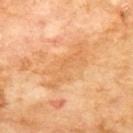Part of a total-body skin-imaging series; this lesion was reviewed on a skin check and was not flagged for biopsy. This image is a 15 mm lesion crop taken from a total-body photograph. From the upper back. A male subject in their 70s. Automated image analysis of the tile measured a lesion color around L≈65 a*≈23 b*≈43 in CIELAB and a lesion-to-skin contrast of about 5 (normalized; higher = more distinct). And it measured a border-irregularity index near 7.5/10, internal color variation of about 2 on a 0–10 scale, and peripheral color asymmetry of about 0.5. Imaged with cross-polarized lighting. The lesion's longest dimension is about 7 mm.